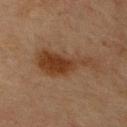Captured during whole-body skin photography for melanoma surveillance; the lesion was not biopsied.
A male patient in their mid-60s.
A roughly 15 mm field-of-view crop from a total-body skin photograph.
On the upper back.
About 8 mm across.